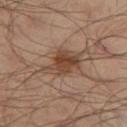Impression: The lesion was tiled from a total-body skin photograph and was not biopsied. Acquisition and patient details: From the left thigh. A 15 mm close-up tile from a total-body photography series done for melanoma screening. The subject is a male in their mid- to late 60s.